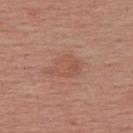Imaged during a routine full-body skin examination; the lesion was not biopsied and no histopathology is available.
Imaged with white-light lighting.
The subject is a female roughly 55 years of age.
A region of skin cropped from a whole-body photographic capture, roughly 15 mm wide.
The recorded lesion diameter is about 4.5 mm.
Located on the upper back.